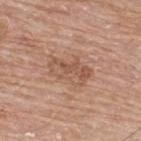Q: Was a biopsy performed?
A: catalogued during a skin exam; not biopsied
Q: Automated lesion metrics?
A: an area of roughly 9.5 mm², an eccentricity of roughly 0.85, and a shape-asymmetry score of about 0.25 (0 = symmetric)
Q: Patient demographics?
A: male, aged around 65
Q: Where on the body is the lesion?
A: the upper back
Q: What kind of image is this?
A: 15 mm crop, total-body photography